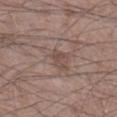workup: imaged on a skin check; not biopsied
subject: male, in their mid- to late 50s
anatomic site: the right lower leg
acquisition: ~15 mm crop, total-body skin-cancer survey